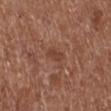{
  "biopsy_status": "not biopsied; imaged during a skin examination"
}